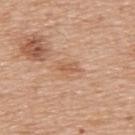follow-up = no biopsy performed (imaged during a skin exam) | patient = male, aged approximately 70 | TBP lesion metrics = a lesion color around L≈59 a*≈22 b*≈34 in CIELAB and a normalized lesion–skin contrast near 5.5; a detector confidence of about 100 out of 100 that the crop contains a lesion | illumination = white-light illumination | acquisition = ~15 mm tile from a whole-body skin photo | anatomic site = the upper back | lesion size = ≈2.5 mm.